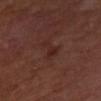Recorded during total-body skin imaging; not selected for excision or biopsy.
A female patient, approximately 55 years of age.
Imaged with cross-polarized lighting.
A lesion tile, about 15 mm wide, cut from a 3D total-body photograph.
Located on the left forearm.
About 3.5 mm across.